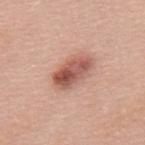Notes:
• biopsy status — total-body-photography surveillance lesion; no biopsy
• lighting — white-light
• size — ≈5 mm
• automated metrics — a nevus-likeness score of about 95/100 and a detector confidence of about 100 out of 100 that the crop contains a lesion
• patient — female, aged 58–62
• location — the mid back
• acquisition — 15 mm crop, total-body photography